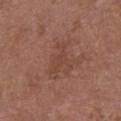This lesion was catalogued during total-body skin photography and was not selected for biopsy.
About 4 mm across.
The lesion is located on the chest.
A female patient, aged approximately 65.
This is a white-light tile.
The total-body-photography lesion software estimated a footprint of about 4 mm², an outline eccentricity of about 0.95 (0 = round, 1 = elongated), and two-axis asymmetry of about 0.5. The software also gave an average lesion color of about L≈43 a*≈22 b*≈27 (CIELAB). The software also gave a border-irregularity index near 6.5/10, a color-variation rating of about 1/10, and a peripheral color-asymmetry measure near 0.5. The software also gave a lesion-detection confidence of about 100/100.
A 15 mm crop from a total-body photograph taken for skin-cancer surveillance.